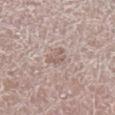Part of a total-body skin-imaging series; this lesion was reviewed on a skin check and was not flagged for biopsy. An algorithmic analysis of the crop reported a shape eccentricity near 0.8 and two-axis asymmetry of about 0.5. It also reported border irregularity of about 5 on a 0–10 scale and a within-lesion color-variation index near 0.5/10. The lesion is located on the leg. Cropped from a whole-body photographic skin survey; the tile spans about 15 mm. This is a white-light tile. A male subject, approximately 65 years of age.